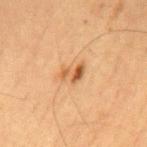biopsy_status: not biopsied; imaged during a skin examination
image:
  source: total-body photography crop
  field_of_view_mm: 15
site: mid back
patient:
  sex: male
  age_approx: 65
automated_metrics:
  area_mm2_approx: 4.0
  eccentricity: 0.8
  shape_asymmetry: 0.45
  color_variation_0_10: 4.0
  peripheral_color_asymmetry: 1.0
  nevus_likeness_0_100: 85
  lesion_detection_confidence_0_100: 100
lesion_size:
  long_diameter_mm_approx: 3.0
lighting: cross-polarized